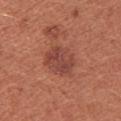Imaged during a routine full-body skin examination; the lesion was not biopsied and no histopathology is available. Automated tile analysis of the lesion measured a footprint of about 11 mm², an eccentricity of roughly 0.55, and two-axis asymmetry of about 0.15. The software also gave a border-irregularity rating of about 2/10, a within-lesion color-variation index near 3.5/10, and radial color variation of about 1.5. A female patient aged 33–37. About 4 mm across. A close-up tile cropped from a whole-body skin photograph, about 15 mm across. This is a white-light tile. Located on the right upper arm.